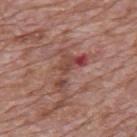| key | value |
|---|---|
| notes | no biopsy performed (imaged during a skin exam) |
| TBP lesion metrics | a border-irregularity index near 9/10, a within-lesion color-variation index near 6/10, and a peripheral color-asymmetry measure near 1; a nevus-likeness score of about 0/100 and a detector confidence of about 90 out of 100 that the crop contains a lesion |
| image | 15 mm crop, total-body photography |
| anatomic site | the mid back |
| lesion diameter | about 5.5 mm |
| subject | male, approximately 70 years of age |